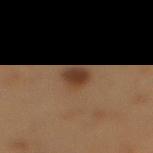  patient:
    sex: male
    age_approx: 55
  lesion_size:
    long_diameter_mm_approx: 3.0
  lighting: cross-polarized
  automated_metrics:
    cielab_L: 30
    cielab_a: 15
    cielab_b: 24
    vs_skin_darker_L: 10.0
    vs_skin_contrast_norm: 9.5
    border_irregularity_0_10: 2.0
    color_variation_0_10: 3.5
    peripheral_color_asymmetry: 1.0
    nevus_likeness_0_100: 95
    lesion_detection_confidence_0_100: 100
  image:
    source: total-body photography crop
    field_of_view_mm: 15
  site: mid back